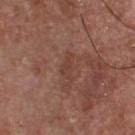The lesion was tiled from a total-body skin photograph and was not biopsied. Automated tile analysis of the lesion measured border irregularity of about 5 on a 0–10 scale and a peripheral color-asymmetry measure near 0.5. Approximately 3.5 mm at its widest. A male patient aged 53 to 57. On the chest. Cropped from a whole-body photographic skin survey; the tile spans about 15 mm. Captured under white-light illumination.